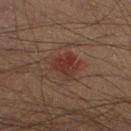follow-up: no biopsy performed (imaged during a skin exam) | TBP lesion metrics: a lesion color around L≈29 a*≈20 b*≈22 in CIELAB, a lesion–skin lightness drop of about 7, and a lesion-to-skin contrast of about 7 (normalized; higher = more distinct); radial color variation of about 1.5; a classifier nevus-likeness of about 15/100 and a lesion-detection confidence of about 100/100 | anatomic site: the left lower leg | diameter: ≈3 mm | patient: male, aged around 40 | image source: total-body-photography crop, ~15 mm field of view | illumination: cross-polarized illumination.